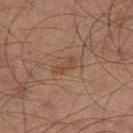Imaged during a routine full-body skin examination; the lesion was not biopsied and no histopathology is available.
Captured under cross-polarized illumination.
Automated image analysis of the tile measured about 6 CIELAB-L* units darker than the surrounding skin and a lesion-to-skin contrast of about 5.5 (normalized; higher = more distinct). The software also gave an automated nevus-likeness rating near 0 out of 100.
The lesion's longest dimension is about 3 mm.
On the right thigh.
Cropped from a whole-body photographic skin survey; the tile spans about 15 mm.
A male subject, aged approximately 70.